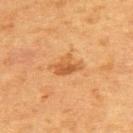<lesion>
  <lesion_size>
    <long_diameter_mm_approx>3.0</long_diameter_mm_approx>
  </lesion_size>
  <patient>
    <sex>female</sex>
    <age_approx>50</age_approx>
  </patient>
  <image>
    <source>total-body photography crop</source>
    <field_of_view_mm>15</field_of_view_mm>
  </image>
  <site>upper back</site>
  <lighting>cross-polarized</lighting>
</lesion>